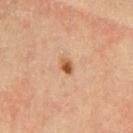patient = female, approximately 60 years of age
illumination = cross-polarized
lesion size = ~2 mm (longest diameter)
image = ~15 mm tile from a whole-body skin photo
location = the left thigh